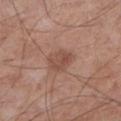Impression: Part of a total-body skin-imaging series; this lesion was reviewed on a skin check and was not flagged for biopsy. Acquisition and patient details: The lesion is on the left lower leg. Imaged with white-light lighting. A male patient approximately 55 years of age. This image is a 15 mm lesion crop taken from a total-body photograph.